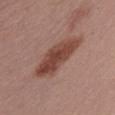Q: Was this lesion biopsied?
A: no biopsy performed (imaged during a skin exam)
Q: Lesion location?
A: the left upper arm
Q: How large is the lesion?
A: ≈6 mm
Q: Who is the patient?
A: female, about 50 years old
Q: How was this image acquired?
A: ~15 mm crop, total-body skin-cancer survey
Q: Illumination type?
A: white-light illumination